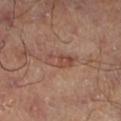The lesion was photographed on a routine skin check and not biopsied; there is no pathology result.